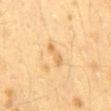| field | value |
|---|---|
| follow-up | imaged on a skin check; not biopsied |
| subject | female, aged approximately 40 |
| size | ≈3.5 mm |
| body site | the mid back |
| image-analysis metrics | a border-irregularity index near 4.5/10, internal color variation of about 0 on a 0–10 scale, and a peripheral color-asymmetry measure near 0; an automated nevus-likeness rating near 0 out of 100 and lesion-presence confidence of about 100/100 |
| tile lighting | cross-polarized |
| acquisition | total-body-photography crop, ~15 mm field of view |